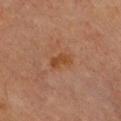Context: A region of skin cropped from a whole-body photographic capture, roughly 15 mm wide. Approximately 3 mm at its widest. Located on the chest. Imaged with cross-polarized lighting. A female patient roughly 65 years of age. Automated tile analysis of the lesion measured a shape eccentricity near 0.85 and a shape-asymmetry score of about 0.35 (0 = symmetric). The analysis additionally found a lesion color around L≈38 a*≈21 b*≈32 in CIELAB, about 7 CIELAB-L* units darker than the surrounding skin, and a normalized border contrast of about 8.